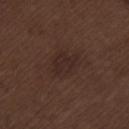Clinical impression:
This lesion was catalogued during total-body skin photography and was not selected for biopsy.
Image and clinical context:
Automated tile analysis of the lesion measured a border-irregularity index near 3/10, a color-variation rating of about 2/10, and radial color variation of about 0.5. Captured under white-light illumination. From the left thigh. A close-up tile cropped from a whole-body skin photograph, about 15 mm across. A male patient about 70 years old. Longest diameter approximately 4.5 mm.